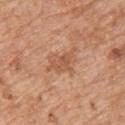Captured during whole-body skin photography for melanoma surveillance; the lesion was not biopsied.
The lesion is on the upper back.
Approximately 4.5 mm at its widest.
A 15 mm crop from a total-body photograph taken for skin-cancer surveillance.
The tile uses white-light illumination.
A male subject in their 60s.